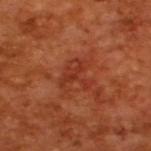The lesion was tiled from a total-body skin photograph and was not biopsied. Cropped from a total-body skin-imaging series; the visible field is about 15 mm. A male subject about 65 years old.